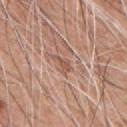biopsy_status: not biopsied; imaged during a skin examination
image:
  source: total-body photography crop
  field_of_view_mm: 15
patient:
  sex: male
  age_approx: 60
automated_metrics:
  area_mm2_approx: 2.5
  eccentricity: 0.9
  shape_asymmetry: 0.5
  vs_skin_darker_L: 9.0
  vs_skin_contrast_norm: 6.0
  border_irregularity_0_10: 5.5
  color_variation_0_10: 0.0
  peripheral_color_asymmetry: 0.0
  nevus_likeness_0_100: 0
  lesion_detection_confidence_0_100: 65
lighting: white-light
site: chest
lesion_size:
  long_diameter_mm_approx: 3.0